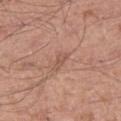Imaged with white-light lighting. The lesion's longest dimension is about 2.5 mm. The lesion-visualizer software estimated a footprint of about 3 mm², an eccentricity of roughly 0.85, and two-axis asymmetry of about 0.35. It also reported border irregularity of about 3.5 on a 0–10 scale and a peripheral color-asymmetry measure near 0. The lesion is located on the leg. A region of skin cropped from a whole-body photographic capture, roughly 15 mm wide. The subject is a male aged around 55.